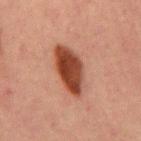The lesion was photographed on a routine skin check and not biopsied; there is no pathology result. Cropped from a total-body skin-imaging series; the visible field is about 15 mm. From the abdomen. Automated tile analysis of the lesion measured internal color variation of about 4.5 on a 0–10 scale. The software also gave an automated nevus-likeness rating near 100 out of 100. The patient is a male about 65 years old. The tile uses cross-polarized illumination. Measured at roughly 6 mm in maximum diameter.